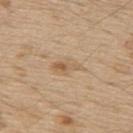Clinical impression:
Imaged during a routine full-body skin examination; the lesion was not biopsied and no histopathology is available.
Image and clinical context:
Located on the upper back. A male patient approximately 70 years of age. A 15 mm close-up tile from a total-body photography series done for melanoma screening.